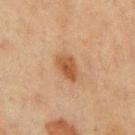The lesion was tiled from a total-body skin photograph and was not biopsied.
The recorded lesion diameter is about 3.5 mm.
A male subject about 70 years old.
A region of skin cropped from a whole-body photographic capture, roughly 15 mm wide.
Located on the mid back.
This is a cross-polarized tile.